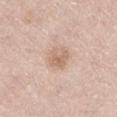follow-up — total-body-photography surveillance lesion; no biopsy
image source — 15 mm crop, total-body photography
location — the leg
subject — male, aged approximately 70
lesion size — about 2.5 mm